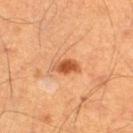workup: catalogued during a skin exam; not biopsied | patient: male, about 60 years old | illumination: cross-polarized | image source: total-body-photography crop, ~15 mm field of view | lesion diameter: about 3.5 mm | site: the right thigh.